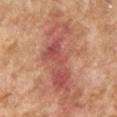notes — catalogued during a skin exam; not biopsied
subject — female, aged 58–62
image source — ~15 mm crop, total-body skin-cancer survey
tile lighting — cross-polarized
lesion diameter — ~10.5 mm (longest diameter)
site — the left lower leg